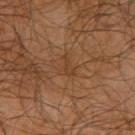Q: Was this lesion biopsied?
A: no biopsy performed (imaged during a skin exam)
Q: What is the imaging modality?
A: 15 mm crop, total-body photography
Q: Who is the patient?
A: male, approximately 65 years of age
Q: Where on the body is the lesion?
A: the right upper arm